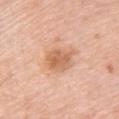The lesion was photographed on a routine skin check and not biopsied; there is no pathology result. The lesion-visualizer software estimated a footprint of about 10 mm², an outline eccentricity of about 0.7 (0 = round, 1 = elongated), and two-axis asymmetry of about 0.2. The software also gave a mean CIELAB color near L≈65 a*≈23 b*≈35 and about 10 CIELAB-L* units darker than the surrounding skin. The analysis additionally found radial color variation of about 1. And it measured a classifier nevus-likeness of about 40/100 and a lesion-detection confidence of about 100/100. A 15 mm crop from a total-body photograph taken for skin-cancer surveillance. The subject is a female aged 48 to 52. On the upper back. About 4.5 mm across. Imaged with white-light lighting.